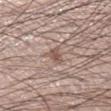notes: total-body-photography surveillance lesion; no biopsy | lighting: white-light illumination | patient: male, in their 50s | site: the right lower leg | image: 15 mm crop, total-body photography.